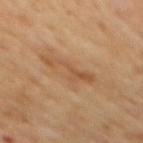Recorded during total-body skin imaging; not selected for excision or biopsy.